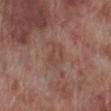Clinical impression: The lesion was tiled from a total-body skin photograph and was not biopsied. Image and clinical context: Located on the right lower leg. The recorded lesion diameter is about 3 mm. A region of skin cropped from a whole-body photographic capture, roughly 15 mm wide. The patient is a male in their 70s. Automated tile analysis of the lesion measured a footprint of about 5 mm², an eccentricity of roughly 0.75, and a shape-asymmetry score of about 0.25 (0 = symmetric). The software also gave a mean CIELAB color near L≈45 a*≈20 b*≈23 and roughly 6 lightness units darker than nearby skin. And it measured a within-lesion color-variation index near 2.5/10 and peripheral color asymmetry of about 1. The software also gave an automated nevus-likeness rating near 0 out of 100 and a detector confidence of about 100 out of 100 that the crop contains a lesion. This is a white-light tile.